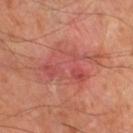Q: Was this lesion biopsied?
A: total-body-photography surveillance lesion; no biopsy
Q: Who is the patient?
A: male, approximately 70 years of age
Q: Lesion location?
A: the left lower leg
Q: What kind of image is this?
A: 15 mm crop, total-body photography
Q: What lighting was used for the tile?
A: cross-polarized
Q: How large is the lesion?
A: ≈7.5 mm